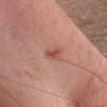notes — total-body-photography surveillance lesion; no biopsy | subject — female, aged 38 to 42 | TBP lesion metrics — two-axis asymmetry of about 0.3; a border-irregularity rating of about 3/10, a within-lesion color-variation index near 1.5/10, and a peripheral color-asymmetry measure near 0.5 | tile lighting — white-light illumination | image — ~15 mm tile from a whole-body skin photo | lesion size — about 3 mm | location — the head or neck.